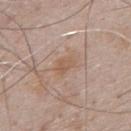The lesion was photographed on a routine skin check and not biopsied; there is no pathology result.
From the chest.
Cropped from a total-body skin-imaging series; the visible field is about 15 mm.
A male subject, aged around 55.
The tile uses white-light illumination.
Automated tile analysis of the lesion measured a lesion area of about 4.5 mm², an outline eccentricity of about 0.75 (0 = round, 1 = elongated), and two-axis asymmetry of about 0.25. And it measured border irregularity of about 2.5 on a 0–10 scale.